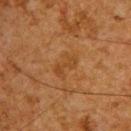{
  "biopsy_status": "not biopsied; imaged during a skin examination",
  "lighting": "cross-polarized",
  "image": {
    "source": "total-body photography crop",
    "field_of_view_mm": 15
  },
  "site": "upper back",
  "patient": {
    "sex": "male",
    "age_approx": 65
  },
  "lesion_size": {
    "long_diameter_mm_approx": 3.5
  }
}